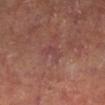{
  "biopsy_status": "not biopsied; imaged during a skin examination"
}